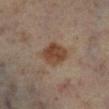Impression: The lesion was tiled from a total-body skin photograph and was not biopsied. Image and clinical context: The lesion is located on the left lower leg. A female patient aged 48 to 52. Cropped from a total-body skin-imaging series; the visible field is about 15 mm. This is a cross-polarized tile.